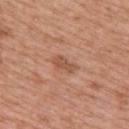Notes:
• workup · total-body-photography surveillance lesion; no biopsy
• tile lighting · white-light
• imaging modality · total-body-photography crop, ~15 mm field of view
• lesion diameter · about 3 mm
• location · the upper back
• patient · female, aged 38 to 42